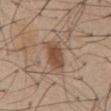Q: Was a biopsy performed?
A: total-body-photography surveillance lesion; no biopsy
Q: What is the imaging modality?
A: 15 mm crop, total-body photography
Q: Who is the patient?
A: male, in their mid- to late 50s
Q: Lesion size?
A: ~3.5 mm (longest diameter)
Q: What is the anatomic site?
A: the abdomen